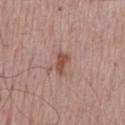| feature | finding |
|---|---|
| follow-up | no biopsy performed (imaged during a skin exam) |
| lesion diameter | ~3 mm (longest diameter) |
| image source | ~15 mm tile from a whole-body skin photo |
| tile lighting | white-light illumination |
| subject | male, aged around 65 |
| site | the mid back |
| TBP lesion metrics | a mean CIELAB color near L≈51 a*≈22 b*≈26, a lesion–skin lightness drop of about 10, and a lesion-to-skin contrast of about 8 (normalized; higher = more distinct); border irregularity of about 3 on a 0–10 scale and a peripheral color-asymmetry measure near 0.5 |